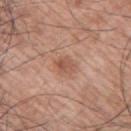A lesion tile, about 15 mm wide, cut from a 3D total-body photograph. Imaged with white-light lighting. The total-body-photography lesion software estimated a footprint of about 4 mm², an outline eccentricity of about 0.65 (0 = round, 1 = elongated), and a shape-asymmetry score of about 0.25 (0 = symmetric). The analysis additionally found a border-irregularity rating of about 2/10, a color-variation rating of about 3/10, and a peripheral color-asymmetry measure near 1. A male patient aged around 70. The lesion is on the left arm.